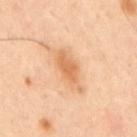Q: What lighting was used for the tile?
A: cross-polarized illumination
Q: How was this image acquired?
A: ~15 mm tile from a whole-body skin photo
Q: Where on the body is the lesion?
A: the mid back
Q: Lesion size?
A: about 5.5 mm
Q: Who is the patient?
A: male, aged approximately 40